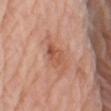– image source · total-body-photography crop, ~15 mm field of view
– subject · male, in their 80s
– location · the arm